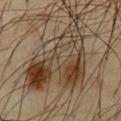Assessment: The lesion was tiled from a total-body skin photograph and was not biopsied. Background: The patient is a male aged 63–67. From the front of the torso. The tile uses cross-polarized illumination. A 15 mm close-up extracted from a 3D total-body photography capture. Measured at roughly 12 mm in maximum diameter. Automated image analysis of the tile measured a lesion area of about 42 mm², a shape eccentricity near 0.85, and a symmetry-axis asymmetry near 0.55. The analysis additionally found a border-irregularity rating of about 8.5/10, internal color variation of about 10 on a 0–10 scale, and peripheral color asymmetry of about 3.5. And it measured a nevus-likeness score of about 0/100 and lesion-presence confidence of about 60/100.